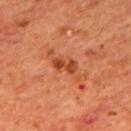patient: male, aged 63–67 | image source: ~15 mm crop, total-body skin-cancer survey | tile lighting: cross-polarized illumination | automated metrics: a shape eccentricity near 0.85 and a symmetry-axis asymmetry near 0.25; a nevus-likeness score of about 0/100 and lesion-presence confidence of about 100/100 | body site: the mid back | lesion size: about 3 mm.